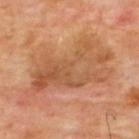biopsy status — no biopsy performed (imaged during a skin exam); patient — male, approximately 65 years of age; lesion size — ~11.5 mm (longest diameter); site — the upper back; TBP lesion metrics — a lesion–skin lightness drop of about 8 and a normalized lesion–skin contrast near 6; image — 15 mm crop, total-body photography.